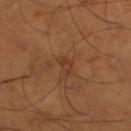Clinical impression: Recorded during total-body skin imaging; not selected for excision or biopsy. Background: This image is a 15 mm lesion crop taken from a total-body photograph. Captured under cross-polarized illumination. A male patient in their mid-50s. The lesion is located on the right thigh.